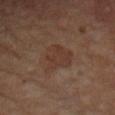About 4 mm across.
A female patient, aged 68–72.
Imaged with cross-polarized lighting.
Located on the left forearm.
A 15 mm crop from a total-body photograph taken for skin-cancer surveillance.